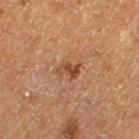Clinical impression:
Part of a total-body skin-imaging series; this lesion was reviewed on a skin check and was not flagged for biopsy.
Clinical summary:
A male subject, aged around 75. Cropped from a whole-body photographic skin survey; the tile spans about 15 mm. Captured under cross-polarized illumination. The lesion is located on the left thigh. Automated image analysis of the tile measured a lesion area of about 4 mm², an outline eccentricity of about 0.75 (0 = round, 1 = elongated), and a shape-asymmetry score of about 0.4 (0 = symmetric). It also reported an automated nevus-likeness rating near 35 out of 100 and a detector confidence of about 100 out of 100 that the crop contains a lesion. About 2.5 mm across.